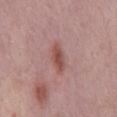| field | value |
|---|---|
| biopsy status | imaged on a skin check; not biopsied |
| lesion size | ≈3.5 mm |
| image-analysis metrics | a shape eccentricity near 0.9 and a shape-asymmetry score of about 0.2 (0 = symmetric); about 10 CIELAB-L* units darker than the surrounding skin and a lesion-to-skin contrast of about 8 (normalized; higher = more distinct); a border-irregularity rating of about 2/10, a color-variation rating of about 3/10, and a peripheral color-asymmetry measure near 1; lesion-presence confidence of about 100/100 |
| image source | ~15 mm tile from a whole-body skin photo |
| anatomic site | the mid back |
| subject | male, aged 58–62 |
| illumination | white-light |